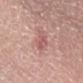Q: Lesion location?
A: the lower back
Q: Illumination type?
A: white-light illumination
Q: Automated lesion metrics?
A: a footprint of about 5.5 mm²; border irregularity of about 3.5 on a 0–10 scale, internal color variation of about 3.5 on a 0–10 scale, and radial color variation of about 1.5; a nevus-likeness score of about 0/100 and a detector confidence of about 100 out of 100 that the crop contains a lesion
Q: What is the imaging modality?
A: 15 mm crop, total-body photography
Q: Who is the patient?
A: male, in their 80s